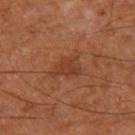This lesion was catalogued during total-body skin photography and was not selected for biopsy. A male patient, aged 63 to 67. On the right thigh. Approximately 3.5 mm at its widest. A 15 mm close-up tile from a total-body photography series done for melanoma screening. This is a cross-polarized tile.